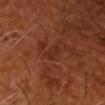follow-up = catalogued during a skin exam; not biopsied | patient = male, aged 63 to 67 | image source = ~15 mm crop, total-body skin-cancer survey | lighting = cross-polarized illumination | lesion diameter = ≈3 mm.